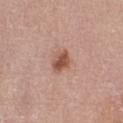Notes:
– follow-up · total-body-photography surveillance lesion; no biopsy